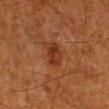Part of a total-body skin-imaging series; this lesion was reviewed on a skin check and was not flagged for biopsy.
A male subject aged approximately 80.
This is a cross-polarized tile.
About 3 mm across.
The lesion is located on the right lower leg.
A close-up tile cropped from a whole-body skin photograph, about 15 mm across.